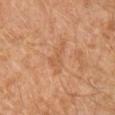Impression:
The lesion was tiled from a total-body skin photograph and was not biopsied.
Context:
A 15 mm close-up extracted from a 3D total-body photography capture. From the left leg. The subject is a male about 30 years old. This is a cross-polarized tile.